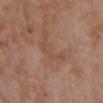follow-up — total-body-photography surveillance lesion; no biopsy
body site — the upper back
patient — female, aged around 35
imaging modality — 15 mm crop, total-body photography
lighting — white-light illumination
size — ≈4.5 mm